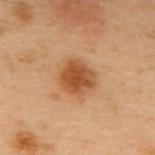Clinical impression:
No biopsy was performed on this lesion — it was imaged during a full skin examination and was not determined to be concerning.
Background:
This image is a 15 mm lesion crop taken from a total-body photograph. The lesion is on the upper back. About 4 mm across. The total-body-photography lesion software estimated a lesion area of about 10 mm² and a shape eccentricity near 0.45. A male subject, aged 53 to 57. Imaged with cross-polarized lighting.